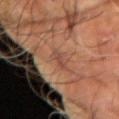biopsy_status: not biopsied; imaged during a skin examination
image:
  source: total-body photography crop
  field_of_view_mm: 15
lesion_size:
  long_diameter_mm_approx: 2.5
lighting: cross-polarized
site: left arm
patient:
  sex: male
  age_approx: 60